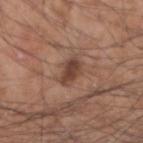Clinical impression:
This lesion was catalogued during total-body skin photography and was not selected for biopsy.
Context:
The lesion is on the right forearm. An algorithmic analysis of the crop reported a lesion color around L≈43 a*≈20 b*≈26 in CIELAB and a normalized border contrast of about 8.5. The analysis additionally found a border-irregularity rating of about 3.5/10, a within-lesion color-variation index near 3.5/10, and peripheral color asymmetry of about 1. Captured under white-light illumination. A close-up tile cropped from a whole-body skin photograph, about 15 mm across. Measured at roughly 4 mm in maximum diameter. A male subject, aged around 55.